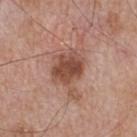The lesion was photographed on a routine skin check and not biopsied; there is no pathology result. A male patient, aged 63 to 67. An algorithmic analysis of the crop reported an area of roughly 9.5 mm², an eccentricity of roughly 0.65, and a symmetry-axis asymmetry near 0.25. The analysis additionally found a color-variation rating of about 3.5/10 and radial color variation of about 1. The software also gave a classifier nevus-likeness of about 5/100 and lesion-presence confidence of about 100/100. The tile uses white-light illumination. This image is a 15 mm lesion crop taken from a total-body photograph. The lesion is on the upper back.